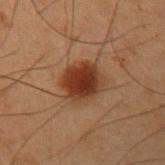The lesion was tiled from a total-body skin photograph and was not biopsied. Cropped from a whole-body photographic skin survey; the tile spans about 15 mm. This is a cross-polarized tile. Approximately 4 mm at its widest. The subject is a male approximately 55 years of age. From the left upper arm.